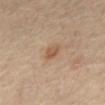<lesion>
  <image>
    <source>total-body photography crop</source>
    <field_of_view_mm>15</field_of_view_mm>
  </image>
  <automated_metrics>
    <shape_asymmetry>0.25</shape_asymmetry>
    <cielab_L>52</cielab_L>
    <cielab_a>18</cielab_a>
    <cielab_b>31</cielab_b>
    <vs_skin_darker_L>8.0</vs_skin_darker_L>
    <vs_skin_contrast_norm>6.5</vs_skin_contrast_norm>
  </automated_metrics>
  <patient>
    <sex>male</sex>
    <age_approx>70</age_approx>
  </patient>
  <site>abdomen</site>
  <lighting>cross-polarized</lighting>
  <lesion_size>
    <long_diameter_mm_approx>2.5</long_diameter_mm_approx>
  </lesion_size>
</lesion>